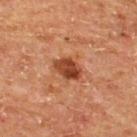notes: imaged on a skin check; not biopsied